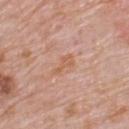follow-up: no biopsy performed (imaged during a skin exam)
location: the upper back
image: total-body-photography crop, ~15 mm field of view
subject: male, aged 73–77
lesion size: about 3 mm